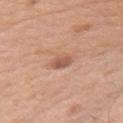The lesion was tiled from a total-body skin photograph and was not biopsied.
A 15 mm close-up extracted from a 3D total-body photography capture.
A female patient in their 50s.
The lesion is located on the right upper arm.